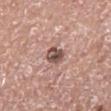{"image": {"source": "total-body photography crop", "field_of_view_mm": 15}, "automated_metrics": {"area_mm2_approx": 5.0, "eccentricity": 0.7, "shape_asymmetry": 0.15, "cielab_L": 50, "cielab_a": 19, "cielab_b": 23, "vs_skin_darker_L": 15.0}, "patient": {"sex": "male", "age_approx": 70}, "lighting": "white-light", "site": "mid back", "lesion_size": {"long_diameter_mm_approx": 3.0}}